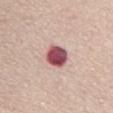<lesion>
  <biopsy_status>not biopsied; imaged during a skin examination</biopsy_status>
  <patient>
    <sex>female</sex>
    <age_approx>70</age_approx>
  </patient>
  <image>
    <source>total-body photography crop</source>
    <field_of_view_mm>15</field_of_view_mm>
  </image>
  <lesion_size>
    <long_diameter_mm_approx>3.0</long_diameter_mm_approx>
  </lesion_size>
  <site>chest</site>
</lesion>